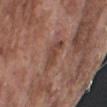biopsy status=total-body-photography surveillance lesion; no biopsy
lighting=white-light
diameter=~3.5 mm (longest diameter)
image-analysis metrics=a lesion–skin lightness drop of about 8 and a normalized lesion–skin contrast near 6.5; border irregularity of about 4 on a 0–10 scale, a color-variation rating of about 2/10, and peripheral color asymmetry of about 1
site=the right upper arm
subject=male, roughly 75 years of age
image=~15 mm tile from a whole-body skin photo